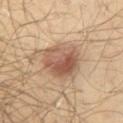Q: Is there a histopathology result?
A: catalogued during a skin exam; not biopsied
Q: What is the lesion's diameter?
A: ~5.5 mm (longest diameter)
Q: Where on the body is the lesion?
A: the chest
Q: How was this image acquired?
A: total-body-photography crop, ~15 mm field of view
Q: Patient demographics?
A: male, about 50 years old